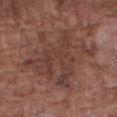Case summary:
- follow-up — total-body-photography surveillance lesion; no biopsy
- image — ~15 mm crop, total-body skin-cancer survey
- lighting — white-light
- body site — the right forearm
- patient — male, aged 73–77
- automated metrics — an outline eccentricity of about 0.5 (0 = round, 1 = elongated); a lesion-detection confidence of about 95/100
- diameter — about 8 mm